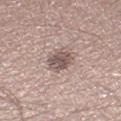The lesion was tiled from a total-body skin photograph and was not biopsied.